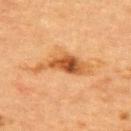Part of a total-body skin-imaging series; this lesion was reviewed on a skin check and was not flagged for biopsy.
The tile uses cross-polarized illumination.
A close-up tile cropped from a whole-body skin photograph, about 15 mm across.
Approximately 6.5 mm at its widest.
A female patient approximately 55 years of age.
On the back.